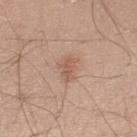  biopsy_status: not biopsied; imaged during a skin examination
  lighting: white-light
  automated_metrics:
    cielab_L: 58
    cielab_a: 20
    cielab_b: 29
    vs_skin_darker_L: 8.0
    vs_skin_contrast_norm: 5.5
    nevus_likeness_0_100: 25
    lesion_detection_confidence_0_100: 100
  lesion_size:
    long_diameter_mm_approx: 3.0
  site: left lower leg
  image:
    source: total-body photography crop
    field_of_view_mm: 15
  patient:
    sex: male
    age_approx: 30